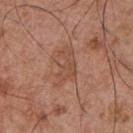This lesion was catalogued during total-body skin photography and was not selected for biopsy. The lesion is located on the front of the torso. Captured under white-light illumination. A 15 mm crop from a total-body photograph taken for skin-cancer surveillance. The recorded lesion diameter is about 4.5 mm. The total-body-photography lesion software estimated a border-irregularity index near 6/10, internal color variation of about 2 on a 0–10 scale, and peripheral color asymmetry of about 0.5. A male patient aged 53–57.